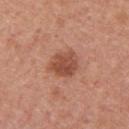Captured during whole-body skin photography for melanoma surveillance; the lesion was not biopsied. Imaged with white-light lighting. A male patient, aged around 30. Located on the upper back. The recorded lesion diameter is about 3.5 mm. A lesion tile, about 15 mm wide, cut from a 3D total-body photograph. An algorithmic analysis of the crop reported an outline eccentricity of about 0.5 (0 = round, 1 = elongated) and two-axis asymmetry of about 0.2. And it measured a within-lesion color-variation index near 3.5/10 and radial color variation of about 1.